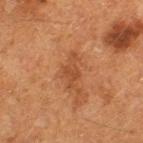Recorded during total-body skin imaging; not selected for excision or biopsy.
The lesion is on the left thigh.
A female patient, approximately 50 years of age.
A 15 mm close-up tile from a total-body photography series done for melanoma screening.
The tile uses cross-polarized illumination.